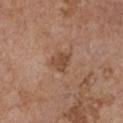Background:
From the chest. The tile uses white-light illumination. The total-body-photography lesion software estimated internal color variation of about 1.5 on a 0–10 scale and peripheral color asymmetry of about 0.5. It also reported a lesion-detection confidence of about 100/100. The recorded lesion diameter is about 2.5 mm. A roughly 15 mm field-of-view crop from a total-body skin photograph. A female subject aged approximately 65.